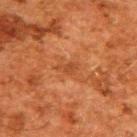image: 15 mm crop, total-body photography | subject: male, about 60 years old | anatomic site: the upper back | lighting: cross-polarized | automated lesion analysis: border irregularity of about 4.5 on a 0–10 scale, a within-lesion color-variation index near 1/10, and peripheral color asymmetry of about 0.5; a classifier nevus-likeness of about 0/100 and a lesion-detection confidence of about 100/100 | diameter: ~2.5 mm (longest diameter).